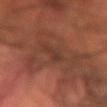Q: Was this lesion biopsied?
A: imaged on a skin check; not biopsied
Q: Automated lesion metrics?
A: a detector confidence of about 85 out of 100 that the crop contains a lesion
Q: Lesion location?
A: the left forearm
Q: What kind of image is this?
A: ~15 mm tile from a whole-body skin photo
Q: What lighting was used for the tile?
A: cross-polarized
Q: Lesion size?
A: ~3 mm (longest diameter)
Q: Who is the patient?
A: male, aged 68 to 72